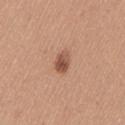biopsy_status: not biopsied; imaged during a skin examination
image:
  source: total-body photography crop
  field_of_view_mm: 15
patient:
  sex: female
  age_approx: 40
automated_metrics:
  color_variation_0_10: 4.5
  peripheral_color_asymmetry: 2.0
lesion_size:
  long_diameter_mm_approx: 3.0
site: left thigh